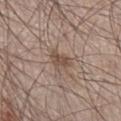| feature | finding |
|---|---|
| notes | imaged on a skin check; not biopsied |
| subject | male, aged 58–62 |
| image | ~15 mm tile from a whole-body skin photo |
| tile lighting | white-light |
| anatomic site | the right lower leg |